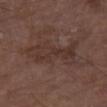| field | value |
|---|---|
| patient | male, aged 73–77 |
| site | the right thigh |
| illumination | white-light |
| automated lesion analysis | a footprint of about 17 mm² and an eccentricity of roughly 0.9; an average lesion color of about L≈33 a*≈16 b*≈21 (CIELAB), a lesion–skin lightness drop of about 6, and a lesion-to-skin contrast of about 6 (normalized; higher = more distinct); a border-irregularity rating of about 6/10, a within-lesion color-variation index near 3/10, and peripheral color asymmetry of about 1; a nevus-likeness score of about 0/100 and lesion-presence confidence of about 95/100 |
| imaging modality | 15 mm crop, total-body photography |
| size | about 7 mm |